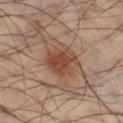Q: Was a biopsy performed?
A: imaged on a skin check; not biopsied
Q: What lighting was used for the tile?
A: cross-polarized
Q: What are the patient's age and sex?
A: male, aged 43–47
Q: What is the anatomic site?
A: the leg
Q: What is the imaging modality?
A: total-body-photography crop, ~15 mm field of view
Q: How large is the lesion?
A: about 4.5 mm